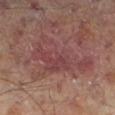notes: imaged on a skin check; not biopsied | site: the left lower leg | image: ~15 mm tile from a whole-body skin photo | patient: male, roughly 70 years of age.